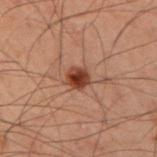Imaged during a routine full-body skin examination; the lesion was not biopsied and no histopathology is available. The subject is a male in their 60s. The lesion is located on the right upper arm. A lesion tile, about 15 mm wide, cut from a 3D total-body photograph.